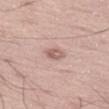Clinical impression:
Recorded during total-body skin imaging; not selected for excision or biopsy.
Clinical summary:
From the left thigh. A region of skin cropped from a whole-body photographic capture, roughly 15 mm wide. A male patient aged 53–57. About 2.5 mm across.